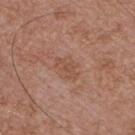{
  "biopsy_status": "not biopsied; imaged during a skin examination",
  "lesion_size": {
    "long_diameter_mm_approx": 3.0
  },
  "image": {
    "source": "total-body photography crop",
    "field_of_view_mm": 15
  },
  "site": "chest",
  "patient": {
    "sex": "male",
    "age_approx": 55
  },
  "lighting": "white-light"
}